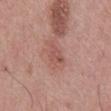The lesion was photographed on a routine skin check and not biopsied; there is no pathology result.